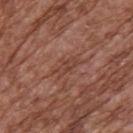Assessment:
No biopsy was performed on this lesion — it was imaged during a full skin examination and was not determined to be concerning.
Background:
Cropped from a total-body skin-imaging series; the visible field is about 15 mm. This is a white-light tile. Located on the upper back. A male subject, in their mid- to late 70s.